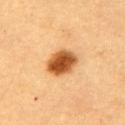A region of skin cropped from a whole-body photographic capture, roughly 15 mm wide. A female subject, approximately 60 years of age. On the back.